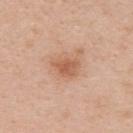The lesion was tiled from a total-body skin photograph and was not biopsied.